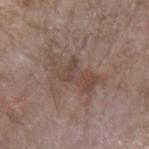Assessment: Imaged during a routine full-body skin examination; the lesion was not biopsied and no histopathology is available. Clinical summary: A lesion tile, about 15 mm wide, cut from a 3D total-body photograph. A female patient, in their mid- to late 70s. The lesion is located on the left forearm. Longest diameter approximately 6.5 mm.